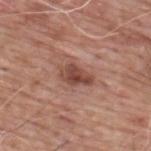Assessment:
Part of a total-body skin-imaging series; this lesion was reviewed on a skin check and was not flagged for biopsy.
Image and clinical context:
The lesion is located on the upper back. This image is a 15 mm lesion crop taken from a total-body photograph. This is a white-light tile. About 3.5 mm across. A male patient approximately 60 years of age.